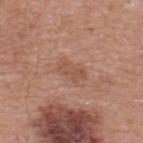Assessment: Imaged during a routine full-body skin examination; the lesion was not biopsied and no histopathology is available. Image and clinical context: Located on the upper back. About 3 mm across. A male patient in their mid- to late 50s. A 15 mm crop from a total-body photograph taken for skin-cancer surveillance. The tile uses white-light illumination.